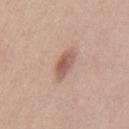<lesion>
<biopsy_status>not biopsied; imaged during a skin examination</biopsy_status>
<lesion_size>
  <long_diameter_mm_approx>3.5</long_diameter_mm_approx>
</lesion_size>
<lighting>white-light</lighting>
<image>
  <source>total-body photography crop</source>
  <field_of_view_mm>15</field_of_view_mm>
</image>
<site>mid back</site>
<patient>
  <sex>male</sex>
  <age_approx>45</age_approx>
</patient>
</lesion>